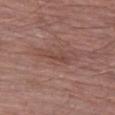The patient is a male aged around 75. An algorithmic analysis of the crop reported a lesion area of about 4 mm², a shape eccentricity near 0.95, and a shape-asymmetry score of about 0.3 (0 = symmetric). The analysis additionally found a mean CIELAB color near L≈45 a*≈21 b*≈23, roughly 7 lightness units darker than nearby skin, and a normalized border contrast of about 5.5. The analysis additionally found a color-variation rating of about 1/10. A 15 mm close-up tile from a total-body photography series done for melanoma screening. The lesion's longest dimension is about 3.5 mm. Located on the left thigh. The tile uses white-light illumination.